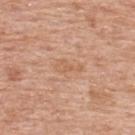patient:
  sex: male
  age_approx: 60
site: upper back
automated_metrics:
  eccentricity: 0.9
  border_irregularity_0_10: 5.5
  peripheral_color_asymmetry: 0.5
lesion_size:
  long_diameter_mm_approx: 3.5
image:
  source: total-body photography crop
  field_of_view_mm: 15